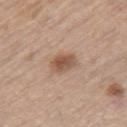Impression:
Recorded during total-body skin imaging; not selected for excision or biopsy.
Image and clinical context:
The patient is a male in their 70s. This image is a 15 mm lesion crop taken from a total-body photograph. The lesion is on the right thigh.